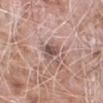lesion size: ≈3 mm | image source: total-body-photography crop, ~15 mm field of view | location: the left forearm | subject: male, approximately 75 years of age.